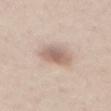biopsy_status: not biopsied; imaged during a skin examination
lighting: white-light
patient:
  sex: male
  age_approx: 35
image:
  source: total-body photography crop
  field_of_view_mm: 15
automated_metrics:
  cielab_L: 62
  cielab_a: 16
  cielab_b: 25
  vs_skin_darker_L: 12.0
  vs_skin_contrast_norm: 7.5
  border_irregularity_0_10: 2.0
  color_variation_0_10: 3.5
  peripheral_color_asymmetry: 1.0
  nevus_likeness_0_100: 85
  lesion_detection_confidence_0_100: 100
site: abdomen
lesion_size:
  long_diameter_mm_approx: 4.0